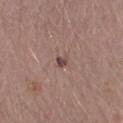Assessment:
Captured during whole-body skin photography for melanoma surveillance; the lesion was not biopsied.
Context:
A 15 mm close-up extracted from a 3D total-body photography capture. The patient is a male about 30 years old. The lesion-visualizer software estimated a footprint of about 1.5 mm², an outline eccentricity of about 0.4 (0 = round, 1 = elongated), and two-axis asymmetry of about 0.35. The software also gave a lesion-to-skin contrast of about 9.5 (normalized; higher = more distinct). The software also gave a detector confidence of about 100 out of 100 that the crop contains a lesion. Approximately 1.5 mm at its widest.